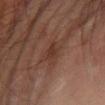acquisition: ~15 mm crop, total-body skin-cancer survey; subject: male, aged approximately 65; site: the left forearm.